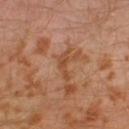Impression: Captured during whole-body skin photography for melanoma surveillance; the lesion was not biopsied. Clinical summary: A 15 mm close-up extracted from a 3D total-body photography capture. From the left leg. Measured at roughly 3.5 mm in maximum diameter. The patient is a male aged around 30. This is a cross-polarized tile.